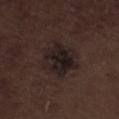<lesion>
<biopsy_status>not biopsied; imaged during a skin examination</biopsy_status>
</lesion>